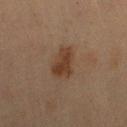A 15 mm close-up tile from a total-body photography series done for melanoma screening.
Longest diameter approximately 3.5 mm.
From the leg.
A female subject, aged approximately 70.
The total-body-photography lesion software estimated a within-lesion color-variation index near 3/10.